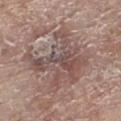notes: catalogued during a skin exam; not biopsied | lighting: white-light | body site: the left lower leg | TBP lesion metrics: a footprint of about 28 mm², a shape eccentricity near 0.55, and two-axis asymmetry of about 0.45; a lesion color around L≈50 a*≈17 b*≈20 in CIELAB, roughly 9 lightness units darker than nearby skin, and a lesion-to-skin contrast of about 7 (normalized; higher = more distinct); a border-irregularity rating of about 7.5/10 and a color-variation rating of about 7/10 | image: 15 mm crop, total-body photography | patient: female, aged approximately 85 or older | lesion diameter: about 7 mm.